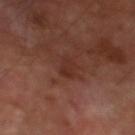Captured during whole-body skin photography for melanoma surveillance; the lesion was not biopsied. The recorded lesion diameter is about 2.5 mm. The patient is a male aged 68 to 72. Captured under cross-polarized illumination. A 15 mm close-up tile from a total-body photography series done for melanoma screening. The lesion is on the leg.